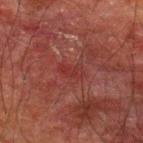The lesion is on the back. Captured under cross-polarized illumination. Measured at roughly 2.5 mm in maximum diameter. The patient is a male aged 58 to 62. An algorithmic analysis of the crop reported border irregularity of about 4 on a 0–10 scale. A close-up tile cropped from a whole-body skin photograph, about 15 mm across.